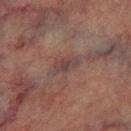follow-up: imaged on a skin check; not biopsied
acquisition: ~15 mm crop, total-body skin-cancer survey
body site: the right lower leg
patient: male, approximately 75 years of age